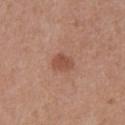Assessment: Imaged during a routine full-body skin examination; the lesion was not biopsied and no histopathology is available. Clinical summary: Approximately 2.5 mm at its widest. A region of skin cropped from a whole-body photographic capture, roughly 15 mm wide. Captured under white-light illumination. The lesion is on the upper back. The patient is a female about 40 years old.